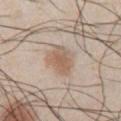| field | value |
|---|---|
| biopsy status | no biopsy performed (imaged during a skin exam) |
| acquisition | total-body-photography crop, ~15 mm field of view |
| location | the chest |
| lesion size | ~4 mm (longest diameter) |
| patient | male, in their mid-40s |
| illumination | white-light |